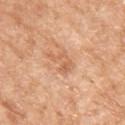On the arm.
Automated image analysis of the tile measured a mean CIELAB color near L≈63 a*≈23 b*≈37 and a lesion–skin lightness drop of about 9. It also reported a border-irregularity rating of about 6/10, a color-variation rating of about 3.5/10, and peripheral color asymmetry of about 1.5.
A female patient, roughly 75 years of age.
A close-up tile cropped from a whole-body skin photograph, about 15 mm across.